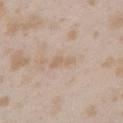Q: Was this lesion biopsied?
A: imaged on a skin check; not biopsied
Q: What kind of image is this?
A: 15 mm crop, total-body photography
Q: What is the anatomic site?
A: the left upper arm
Q: Who is the patient?
A: female, about 25 years old
Q: Illumination type?
A: white-light illumination
Q: What is the lesion's diameter?
A: ≈3 mm